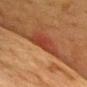The lesion was tiled from a total-body skin photograph and was not biopsied. A 15 mm close-up extracted from a 3D total-body photography capture. The tile uses cross-polarized illumination. A female subject, aged 53 to 57. The recorded lesion diameter is about 4 mm. The lesion is located on the chest. An algorithmic analysis of the crop reported an area of roughly 9 mm², an outline eccentricity of about 0.65 (0 = round, 1 = elongated), and two-axis asymmetry of about 0.35. It also reported a classifier nevus-likeness of about 20/100 and lesion-presence confidence of about 65/100.